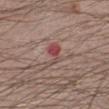Captured during whole-body skin photography for melanoma surveillance; the lesion was not biopsied.
The lesion is located on the leg.
About 3 mm across.
A roughly 15 mm field-of-view crop from a total-body skin photograph.
The patient is a male about 35 years old.